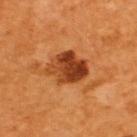The lesion was photographed on a routine skin check and not biopsied; there is no pathology result. The lesion is located on the upper back. Approximately 4.5 mm at its widest. The patient is a male about 60 years old. Cropped from a whole-body photographic skin survey; the tile spans about 15 mm.